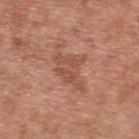The lesion was tiled from a total-body skin photograph and was not biopsied.
A male subject, about 70 years old.
The lesion is located on the upper back.
A roughly 15 mm field-of-view crop from a total-body skin photograph.